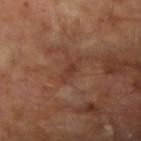Assessment:
The lesion was photographed on a routine skin check and not biopsied; there is no pathology result.
Context:
The total-body-photography lesion software estimated a footprint of about 3 mm², a shape eccentricity near 0.95, and two-axis asymmetry of about 0.25. The analysis additionally found internal color variation of about 0 on a 0–10 scale. The software also gave an automated nevus-likeness rating near 0 out of 100 and a detector confidence of about 100 out of 100 that the crop contains a lesion. A lesion tile, about 15 mm wide, cut from a 3D total-body photograph. The patient is a male aged 63–67. Longest diameter approximately 3.5 mm. From the right upper arm. The tile uses cross-polarized illumination.